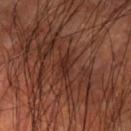No biopsy was performed on this lesion — it was imaged during a full skin examination and was not determined to be concerning. The patient is a male aged 58–62. From the left upper arm. A close-up tile cropped from a whole-body skin photograph, about 15 mm across.